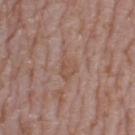Part of a total-body skin-imaging series; this lesion was reviewed on a skin check and was not flagged for biopsy.
Approximately 3.5 mm at its widest.
A female subject approximately 60 years of age.
The tile uses white-light illumination.
A roughly 15 mm field-of-view crop from a total-body skin photograph.
Located on the left thigh.